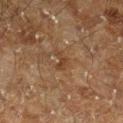Context:
Automated tile analysis of the lesion measured an outline eccentricity of about 0.85 (0 = round, 1 = elongated) and a shape-asymmetry score of about 0.45 (0 = symmetric). It also reported a border-irregularity rating of about 5/10 and a peripheral color-asymmetry measure near 0. The tile uses cross-polarized illumination. A 15 mm close-up extracted from a 3D total-body photography capture. The lesion is on the right lower leg. Longest diameter approximately 3 mm. A male subject aged approximately 60.